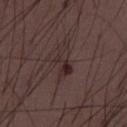The lesion was photographed on a routine skin check and not biopsied; there is no pathology result. A lesion tile, about 15 mm wide, cut from a 3D total-body photograph. About 3.5 mm across. A male patient, aged around 50. The tile uses white-light illumination.